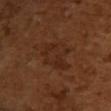{
  "biopsy_status": "not biopsied; imaged during a skin examination",
  "image": {
    "source": "total-body photography crop",
    "field_of_view_mm": 15
  },
  "site": "upper back",
  "patient": {
    "sex": "female",
    "age_approx": 55
  }
}